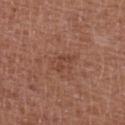patient: male, about 75 years old | body site: the left lower leg | imaging modality: ~15 mm tile from a whole-body skin photo | lighting: white-light | lesion size: about 3.5 mm.